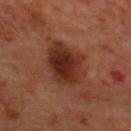{
  "biopsy_status": "not biopsied; imaged during a skin examination",
  "image": {
    "source": "total-body photography crop",
    "field_of_view_mm": 15
  },
  "lighting": "cross-polarized",
  "site": "upper back",
  "patient": {
    "sex": "male",
    "age_approx": 70
  },
  "lesion_size": {
    "long_diameter_mm_approx": 5.5
  }
}